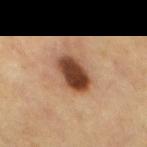Recorded during total-body skin imaging; not selected for excision or biopsy. A close-up tile cropped from a whole-body skin photograph, about 15 mm across. The tile uses cross-polarized illumination. The subject is a male aged 63–67. From the chest. About 4.5 mm across.